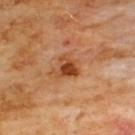Q: Is there a histopathology result?
A: no biopsy performed (imaged during a skin exam)
Q: What are the patient's age and sex?
A: male, aged 58 to 62
Q: How large is the lesion?
A: about 3 mm
Q: Automated lesion metrics?
A: a footprint of about 6 mm², an eccentricity of roughly 0.4, and two-axis asymmetry of about 0.4; an average lesion color of about L≈44 a*≈26 b*≈37 (CIELAB), a lesion–skin lightness drop of about 12, and a lesion-to-skin contrast of about 9 (normalized; higher = more distinct); internal color variation of about 6 on a 0–10 scale
Q: Lesion location?
A: the front of the torso
Q: What is the imaging modality?
A: total-body-photography crop, ~15 mm field of view
Q: How was the tile lit?
A: cross-polarized illumination